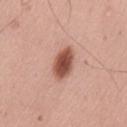Assessment:
This lesion was catalogued during total-body skin photography and was not selected for biopsy.
Context:
The subject is a male aged 38–42. The lesion is located on the lower back. A close-up tile cropped from a whole-body skin photograph, about 15 mm across. Longest diameter approximately 4 mm. Imaged with white-light lighting.